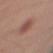lighting: white-light
lesion_size:
  long_diameter_mm_approx: 3.5
image:
  source: total-body photography crop
  field_of_view_mm: 15
patient:
  sex: male
  age_approx: 35
site: left upper arm
automated_metrics:
  area_mm2_approx: 7.5
  eccentricity: 0.65
  color_variation_0_10: 3.5
  peripheral_color_asymmetry: 1.0
  nevus_likeness_0_100: 100
  lesion_detection_confidence_0_100: 100